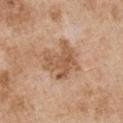workup: no biopsy performed (imaged during a skin exam) | illumination: white-light | image-analysis metrics: a shape eccentricity near 0.25 and a shape-asymmetry score of about 0.4 (0 = symmetric); an average lesion color of about L≈56 a*≈20 b*≈33 (CIELAB), about 10 CIELAB-L* units darker than the surrounding skin, and a normalized border contrast of about 7.5; an automated nevus-likeness rating near 0 out of 100 and a lesion-detection confidence of about 100/100 | body site: the chest | diameter: about 4.5 mm | image source: total-body-photography crop, ~15 mm field of view | subject: male, aged 53 to 57.